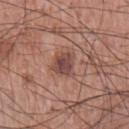Imaged during a routine full-body skin examination; the lesion was not biopsied and no histopathology is available. A 15 mm close-up tile from a total-body photography series done for melanoma screening. On the chest. Captured under white-light illumination. The subject is a male aged 73 to 77. Longest diameter approximately 3 mm.